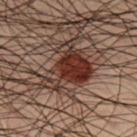The lesion was photographed on a routine skin check and not biopsied; there is no pathology result. Approximately 6 mm at its widest. A 15 mm close-up tile from a total-body photography series done for melanoma screening. Located on the right lower leg. The tile uses cross-polarized illumination. A male patient, aged 48–52.